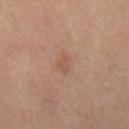biopsy_status: not biopsied; imaged during a skin examination